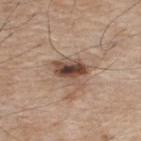biopsy status — no biopsy performed (imaged during a skin exam)
diameter — ~4 mm (longest diameter)
image source — ~15 mm crop, total-body skin-cancer survey
site — the upper back
patient — male, in their mid- to late 70s
image-analysis metrics — an average lesion color of about L≈47 a*≈17 b*≈26 (CIELAB) and about 15 CIELAB-L* units darker than the surrounding skin; a border-irregularity rating of about 4/10 and radial color variation of about 3; a classifier nevus-likeness of about 95/100